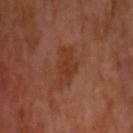Q: Is there a histopathology result?
A: total-body-photography surveillance lesion; no biopsy
Q: Lesion location?
A: the head or neck
Q: Patient demographics?
A: male, in their mid- to late 50s
Q: Lesion size?
A: about 4.5 mm
Q: What did automated image analysis measure?
A: an area of roughly 9.5 mm², an outline eccentricity of about 0.85 (0 = round, 1 = elongated), and two-axis asymmetry of about 0.35; a lesion color around L≈37 a*≈24 b*≈31 in CIELAB and a normalized border contrast of about 6; a color-variation rating of about 2.5/10; a nevus-likeness score of about 5/100 and a detector confidence of about 100 out of 100 that the crop contains a lesion
Q: Illumination type?
A: cross-polarized
Q: How was this image acquired?
A: ~15 mm tile from a whole-body skin photo